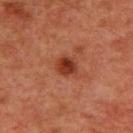{
  "biopsy_status": "not biopsied; imaged during a skin examination",
  "image": {
    "source": "total-body photography crop",
    "field_of_view_mm": 15
  },
  "patient": {
    "sex": "male",
    "age_approx": 60
  },
  "site": "chest"
}